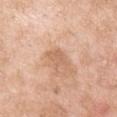notes = total-body-photography surveillance lesion; no biopsy
patient = male, roughly 55 years of age
acquisition = total-body-photography crop, ~15 mm field of view
body site = the right upper arm